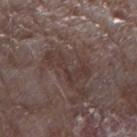Impression:
Imaged during a routine full-body skin examination; the lesion was not biopsied and no histopathology is available.
Context:
The lesion is on the left forearm. The tile uses white-light illumination. Automated tile analysis of the lesion measured a footprint of about 7.5 mm². A male subject aged around 65. A region of skin cropped from a whole-body photographic capture, roughly 15 mm wide.